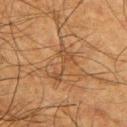Assessment: The lesion was tiled from a total-body skin photograph and was not biopsied. Background: The total-body-photography lesion software estimated a border-irregularity rating of about 9/10 and a peripheral color-asymmetry measure near 0.5. The lesion is on the right upper arm. The lesion's longest dimension is about 5 mm. Imaged with cross-polarized lighting. A male subject aged around 65. This image is a 15 mm lesion crop taken from a total-body photograph.